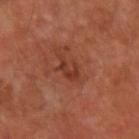workup: total-body-photography surveillance lesion; no biopsy | automated lesion analysis: a lesion area of about 4 mm², an outline eccentricity of about 0.8 (0 = round, 1 = elongated), and a symmetry-axis asymmetry near 0.45; roughly 7 lightness units darker than nearby skin and a normalized lesion–skin contrast near 6.5; a border-irregularity rating of about 6.5/10, a within-lesion color-variation index near 0/10, and peripheral color asymmetry of about 0 | lighting: cross-polarized illumination | imaging modality: ~15 mm tile from a whole-body skin photo | diameter: about 3 mm | patient: male, approximately 50 years of age | anatomic site: the left forearm.